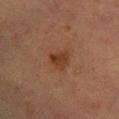Recorded during total-body skin imaging; not selected for excision or biopsy. The tile uses cross-polarized illumination. Approximately 2.5 mm at its widest. The patient is a female about 55 years old. An algorithmic analysis of the crop reported a mean CIELAB color near L≈29 a*≈19 b*≈26, roughly 7 lightness units darker than nearby skin, and a normalized border contrast of about 7.5. And it measured an automated nevus-likeness rating near 50 out of 100 and lesion-presence confidence of about 100/100. A lesion tile, about 15 mm wide, cut from a 3D total-body photograph. From the leg.